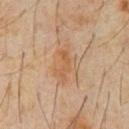{
  "biopsy_status": "not biopsied; imaged during a skin examination",
  "image": {
    "source": "total-body photography crop",
    "field_of_view_mm": 15
  },
  "automated_metrics": {
    "cielab_L": 54,
    "cielab_a": 18,
    "cielab_b": 33,
    "vs_skin_darker_L": 6.0,
    "vs_skin_contrast_norm": 5.5,
    "nevus_likeness_0_100": 10,
    "lesion_detection_confidence_0_100": 100
  },
  "site": "abdomen",
  "lighting": "cross-polarized",
  "patient": {
    "sex": "male",
    "age_approx": 60
  },
  "lesion_size": {
    "long_diameter_mm_approx": 5.0
  }
}